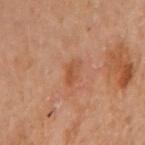biopsy status: no biopsy performed (imaged during a skin exam); site: the arm; acquisition: ~15 mm tile from a whole-body skin photo; lesion size: ~2.5 mm (longest diameter); tile lighting: cross-polarized illumination; patient: female, aged 58–62.